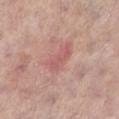The lesion was tiled from a total-body skin photograph and was not biopsied. The tile uses white-light illumination. A female subject in their 60s. From the leg. This image is a 15 mm lesion crop taken from a total-body photograph. The lesion-visualizer software estimated a border-irregularity rating of about 5.5/10 and a color-variation rating of about 0/10. Longest diameter approximately 3 mm.